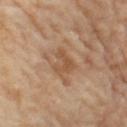Assessment: Recorded during total-body skin imaging; not selected for excision or biopsy. Clinical summary: Located on the left thigh. Automated image analysis of the tile measured an area of roughly 5.5 mm² and two-axis asymmetry of about 0.6. The software also gave a mean CIELAB color near L≈53 a*≈20 b*≈34, roughly 8 lightness units darker than nearby skin, and a normalized lesion–skin contrast near 6.5. And it measured border irregularity of about 6.5 on a 0–10 scale, a color-variation rating of about 1.5/10, and peripheral color asymmetry of about 0.5. The software also gave a classifier nevus-likeness of about 0/100 and a lesion-detection confidence of about 100/100. A female subject in their 70s. A roughly 15 mm field-of-view crop from a total-body skin photograph. The tile uses cross-polarized illumination. The recorded lesion diameter is about 3 mm.